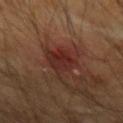<record>
  <biopsy_status>not biopsied; imaged during a skin examination</biopsy_status>
  <image>
    <source>total-body photography crop</source>
    <field_of_view_mm>15</field_of_view_mm>
  </image>
  <lesion_size>
    <long_diameter_mm_approx>4.5</long_diameter_mm_approx>
  </lesion_size>
  <site>arm</site>
  <patient>
    <sex>male</sex>
    <age_approx>55</age_approx>
  </patient>
</record>